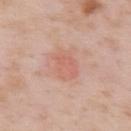| key | value |
|---|---|
| workup | imaged on a skin check; not biopsied |
| lesion size | about 4 mm |
| tile lighting | white-light |
| imaging modality | ~15 mm tile from a whole-body skin photo |
| image-analysis metrics | a mean CIELAB color near L≈62 a*≈24 b*≈29, roughly 6 lightness units darker than nearby skin, and a lesion-to-skin contrast of about 4.5 (normalized; higher = more distinct); a border-irregularity rating of about 1.5/10; a classifier nevus-likeness of about 15/100 |
| patient | male, aged approximately 55 |
| body site | the upper back |